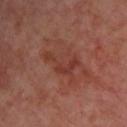Captured during whole-body skin photography for melanoma surveillance; the lesion was not biopsied. Located on the upper back. Measured at roughly 5 mm in maximum diameter. The total-body-photography lesion software estimated a footprint of about 8.5 mm², an eccentricity of roughly 0.85, and a symmetry-axis asymmetry near 0.6. And it measured a mean CIELAB color near L≈37 a*≈25 b*≈26 and roughly 6 lightness units darker than nearby skin. The analysis additionally found a border-irregularity rating of about 8.5/10, internal color variation of about 3 on a 0–10 scale, and peripheral color asymmetry of about 1. It also reported an automated nevus-likeness rating near 0 out of 100 and lesion-presence confidence of about 100/100. Cropped from a total-body skin-imaging series; the visible field is about 15 mm. Captured under cross-polarized illumination. A patient in their mid- to late 50s.